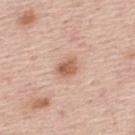| feature | finding |
|---|---|
| workup | imaged on a skin check; not biopsied |
| subject | male, aged around 45 |
| location | the mid back |
| illumination | white-light illumination |
| diameter | about 2.5 mm |
| image | 15 mm crop, total-body photography |